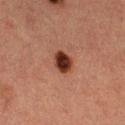Part of a total-body skin-imaging series; this lesion was reviewed on a skin check and was not flagged for biopsy. A female subject, aged approximately 40. Measured at roughly 2.5 mm in maximum diameter. The lesion is located on the left thigh. Cropped from a total-body skin-imaging series; the visible field is about 15 mm.